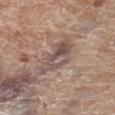notes: no biopsy performed (imaged during a skin exam)
patient: female, approximately 75 years of age
body site: the right thigh
size: about 5 mm
image: ~15 mm crop, total-body skin-cancer survey
illumination: white-light illumination